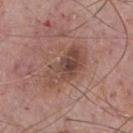Clinical summary: A male subject in their mid- to late 70s. The lesion is located on the chest. The recorded lesion diameter is about 6.5 mm. This is a white-light tile. Cropped from a whole-body photographic skin survey; the tile spans about 15 mm. Automated tile analysis of the lesion measured an area of roughly 15 mm², a shape eccentricity near 0.9, and a shape-asymmetry score of about 0.25 (0 = symmetric). And it measured a border-irregularity index near 3/10, a within-lesion color-variation index near 7.5/10, and peripheral color asymmetry of about 2.5. And it measured a classifier nevus-likeness of about 5/100 and a detector confidence of about 100 out of 100 that the crop contains a lesion.